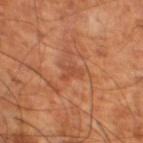biopsy status: total-body-photography surveillance lesion; no biopsy | imaging modality: ~15 mm tile from a whole-body skin photo | patient: male, roughly 60 years of age | lesion size: ~2.5 mm (longest diameter) | location: the leg.